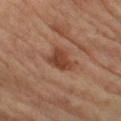biopsy status: no biopsy performed (imaged during a skin exam) | image source: 15 mm crop, total-body photography | patient: female, aged 68 to 72 | automated metrics: an area of roughly 7 mm², an eccentricity of roughly 0.8, and a shape-asymmetry score of about 0.45 (0 = symmetric); a border-irregularity rating of about 4.5/10 and radial color variation of about 0.5; an automated nevus-likeness rating near 85 out of 100 and a detector confidence of about 100 out of 100 that the crop contains a lesion | illumination: cross-polarized | lesion diameter: about 4 mm | anatomic site: the leg.